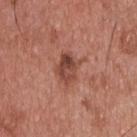Notes:
– workup: imaged on a skin check; not biopsied
– anatomic site: the upper back
– automated lesion analysis: an area of roughly 8 mm², an outline eccentricity of about 0.65 (0 = round, 1 = elongated), and a shape-asymmetry score of about 0.3 (0 = symmetric); a normalized lesion–skin contrast near 8
– imaging modality: 15 mm crop, total-body photography
– lesion diameter: ≈4 mm
– patient: male, aged approximately 55